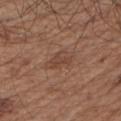Q: Was a biopsy performed?
A: no biopsy performed (imaged during a skin exam)
Q: Lesion location?
A: the left upper arm
Q: What kind of image is this?
A: ~15 mm tile from a whole-body skin photo
Q: What did automated image analysis measure?
A: an area of roughly 6 mm², a shape eccentricity near 0.75, and a symmetry-axis asymmetry near 0.25; an automated nevus-likeness rating near 5 out of 100 and a lesion-detection confidence of about 100/100
Q: Lesion size?
A: ~3.5 mm (longest diameter)
Q: Who is the patient?
A: male, aged 53 to 57
Q: Illumination type?
A: white-light